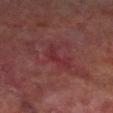Captured during whole-body skin photography for melanoma surveillance; the lesion was not biopsied. An algorithmic analysis of the crop reported an area of roughly 5 mm², an outline eccentricity of about 0.85 (0 = round, 1 = elongated), and a shape-asymmetry score of about 0.7 (0 = symmetric). It also reported a border-irregularity index near 8/10, a color-variation rating of about 1/10, and a peripheral color-asymmetry measure near 0. It also reported a classifier nevus-likeness of about 0/100 and lesion-presence confidence of about 60/100. Imaged with cross-polarized lighting. The lesion is on the leg. A male patient, approximately 70 years of age. A 15 mm close-up extracted from a 3D total-body photography capture. Measured at roughly 4 mm in maximum diameter.